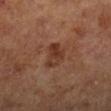This is a cross-polarized tile.
A female subject roughly 65 years of age.
Cropped from a whole-body photographic skin survey; the tile spans about 15 mm.
The recorded lesion diameter is about 3.5 mm.
The lesion is located on the right lower leg.